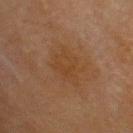| feature | finding |
|---|---|
| workup | imaged on a skin check; not biopsied |
| patient | male, approximately 70 years of age |
| diameter | ≈4 mm |
| body site | the head or neck |
| image source | ~15 mm tile from a whole-body skin photo |
| TBP lesion metrics | a footprint of about 10 mm²; a mean CIELAB color near L≈34 a*≈16 b*≈29, a lesion–skin lightness drop of about 4, and a normalized border contrast of about 4.5 |